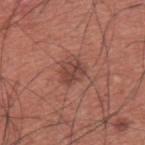{
  "biopsy_status": "not biopsied; imaged during a skin examination",
  "site": "upper back",
  "lighting": "white-light",
  "patient": {
    "sex": "male",
    "age_approx": 35
  },
  "image": {
    "source": "total-body photography crop",
    "field_of_view_mm": 15
  },
  "automated_metrics": {
    "vs_skin_darker_L": 8.0,
    "vs_skin_contrast_norm": 6.5,
    "border_irregularity_0_10": 2.0,
    "peripheral_color_asymmetry": 1.5
  },
  "lesion_size": {
    "long_diameter_mm_approx": 3.0
  }
}